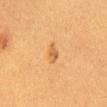{
  "biopsy_status": "not biopsied; imaged during a skin examination",
  "image": {
    "source": "total-body photography crop",
    "field_of_view_mm": 15
  },
  "site": "abdomen",
  "lighting": "cross-polarized",
  "patient": {
    "sex": "female",
    "age_approx": 40
  },
  "automated_metrics": {
    "area_mm2_approx": 2.5,
    "shape_asymmetry": 0.4,
    "border_irregularity_0_10": 3.5,
    "color_variation_0_10": 0.5,
    "peripheral_color_asymmetry": 0.0
  }
}